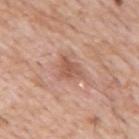Longest diameter approximately 3 mm.
Automated image analysis of the tile measured border irregularity of about 3 on a 0–10 scale and a color-variation rating of about 2.5/10. It also reported a detector confidence of about 100 out of 100 that the crop contains a lesion.
A 15 mm close-up tile from a total-body photography series done for melanoma screening.
Captured under white-light illumination.
Located on the chest.
A male subject, about 60 years old.